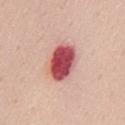<tbp_lesion>
<biopsy_status>not biopsied; imaged during a skin examination</biopsy_status>
<patient>
  <sex>female</sex>
  <age_approx>50</age_approx>
</patient>
<image>
  <source>total-body photography crop</source>
  <field_of_view_mm>15</field_of_view_mm>
</image>
<site>back</site>
</tbp_lesion>